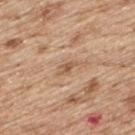Imaged during a routine full-body skin examination; the lesion was not biopsied and no histopathology is available. The lesion is located on the upper back. This is a white-light tile. Measured at roughly 3 mm in maximum diameter. Automated image analysis of the tile measured a mean CIELAB color near L≈57 a*≈19 b*≈33, about 9 CIELAB-L* units darker than the surrounding skin, and a normalized lesion–skin contrast near 6. The software also gave border irregularity of about 4 on a 0–10 scale, a within-lesion color-variation index near 1.5/10, and peripheral color asymmetry of about 0.5. The software also gave a classifier nevus-likeness of about 0/100 and lesion-presence confidence of about 100/100. This image is a 15 mm lesion crop taken from a total-body photograph. A male patient, aged approximately 70.